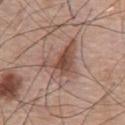{
  "biopsy_status": "not biopsied; imaged during a skin examination",
  "lesion_size": {
    "long_diameter_mm_approx": 5.5
  },
  "image": {
    "source": "total-body photography crop",
    "field_of_view_mm": 15
  },
  "site": "chest",
  "patient": {
    "sex": "male",
    "age_approx": 75
  },
  "lighting": "white-light"
}